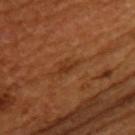Q: Was a biopsy performed?
A: catalogued during a skin exam; not biopsied
Q: What lighting was used for the tile?
A: cross-polarized
Q: What did automated image analysis measure?
A: a lesion area of about 3 mm², an outline eccentricity of about 0.85 (0 = round, 1 = elongated), and two-axis asymmetry of about 0.45; border irregularity of about 4.5 on a 0–10 scale, internal color variation of about 1.5 on a 0–10 scale, and radial color variation of about 0.5
Q: What are the patient's age and sex?
A: female, aged 43–47
Q: Lesion location?
A: the back
Q: What kind of image is this?
A: ~15 mm tile from a whole-body skin photo